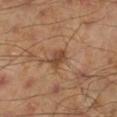follow-up: catalogued during a skin exam; not biopsied
subject: male, aged around 45
size: ~2.5 mm (longest diameter)
lighting: cross-polarized
acquisition: 15 mm crop, total-body photography
site: the left lower leg